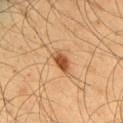notes: total-body-photography surveillance lesion; no biopsy | image: 15 mm crop, total-body photography | automated metrics: a mean CIELAB color near L≈51 a*≈24 b*≈39, a lesion–skin lightness drop of about 14, and a lesion-to-skin contrast of about 9.5 (normalized; higher = more distinct); an automated nevus-likeness rating near 95 out of 100 and a lesion-detection confidence of about 100/100 | lesion size: ~2.5 mm (longest diameter) | illumination: cross-polarized illumination | patient: male, about 55 years old | anatomic site: the right upper arm.